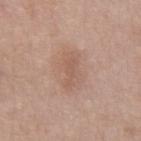| field | value |
|---|---|
| biopsy status | no biopsy performed (imaged during a skin exam) |
| body site | the abdomen |
| diameter | about 3 mm |
| image source | 15 mm crop, total-body photography |
| illumination | white-light |
| subject | male, about 50 years old |